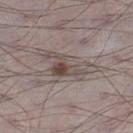• size · ≈7 mm
• acquisition · 15 mm crop, total-body photography
• anatomic site · the right lower leg
• TBP lesion metrics · a mean CIELAB color near L≈49 a*≈12 b*≈17, roughly 9 lightness units darker than nearby skin, and a lesion-to-skin contrast of about 7 (normalized; higher = more distinct); a border-irregularity index near 5.5/10, internal color variation of about 8 on a 0–10 scale, and peripheral color asymmetry of about 2.5; an automated nevus-likeness rating near 70 out of 100 and a detector confidence of about 10 out of 100 that the crop contains a lesion
• patient · male, approximately 70 years of age
• illumination · white-light illumination